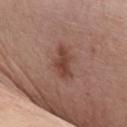  biopsy_status: not biopsied; imaged during a skin examination
  image:
    source: total-body photography crop
    field_of_view_mm: 15
  patient:
    sex: female
    age_approx: 60
  site: chest